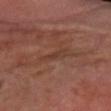Impression: Imaged during a routine full-body skin examination; the lesion was not biopsied and no histopathology is available. Context: The lesion's longest dimension is about 3 mm. A male subject, in their 70s. Imaged with cross-polarized lighting. The lesion is on the right forearm. A 15 mm crop from a total-body photograph taken for skin-cancer surveillance.